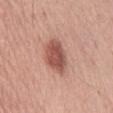Q: Was a biopsy performed?
A: no biopsy performed (imaged during a skin exam)
Q: Patient demographics?
A: male, aged 53–57
Q: What is the anatomic site?
A: the abdomen
Q: What is the imaging modality?
A: ~15 mm crop, total-body skin-cancer survey